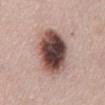<record>
  <site>abdomen</site>
  <image>
    <source>total-body photography crop</source>
    <field_of_view_mm>15</field_of_view_mm>
  </image>
  <patient>
    <sex>male</sex>
    <age_approx>65</age_approx>
  </patient>
</record>